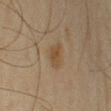follow-up: catalogued during a skin exam; not biopsied
site: the left upper arm
image: 15 mm crop, total-body photography
patient: male, aged around 65